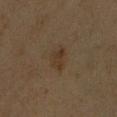Assessment: Imaged during a routine full-body skin examination; the lesion was not biopsied and no histopathology is available. Background: Cropped from a whole-body photographic skin survey; the tile spans about 15 mm. From the right forearm. A female subject aged 53 to 57.